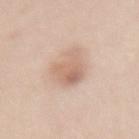| feature | finding |
|---|---|
| subject | female, aged 48–52 |
| illumination | white-light |
| site | the back |
| TBP lesion metrics | a footprint of about 12 mm², a shape eccentricity near 0.8, and a shape-asymmetry score of about 0.3 (0 = symmetric); roughly 10 lightness units darker than nearby skin and a normalized lesion–skin contrast near 6 |
| acquisition | 15 mm crop, total-body photography |